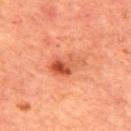Case summary:
* follow-up: imaged on a skin check; not biopsied
* patient: male, aged approximately 65
* lighting: cross-polarized illumination
* size: ≈4.5 mm
* site: the mid back
* imaging modality: 15 mm crop, total-body photography